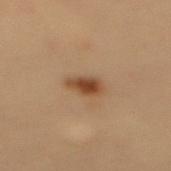Captured during whole-body skin photography for melanoma surveillance; the lesion was not biopsied. This is a cross-polarized tile. The lesion is located on the mid back. A region of skin cropped from a whole-body photographic capture, roughly 15 mm wide. A female subject, aged 38 to 42. The recorded lesion diameter is about 3 mm.